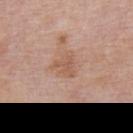{
  "biopsy_status": "not biopsied; imaged during a skin examination",
  "automated_metrics": {
    "area_mm2_approx": 3.5,
    "eccentricity": 0.55,
    "cielab_L": 56,
    "cielab_a": 22,
    "cielab_b": 30,
    "vs_skin_darker_L": 7.0,
    "vs_skin_contrast_norm": 5.5
  },
  "patient": {
    "sex": "male",
    "age_approx": 75
  },
  "image": {
    "source": "total-body photography crop",
    "field_of_view_mm": 15
  },
  "lesion_size": {
    "long_diameter_mm_approx": 2.5
  },
  "site": "mid back",
  "lighting": "white-light"
}